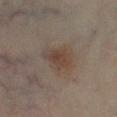Q: Was a biopsy performed?
A: catalogued during a skin exam; not biopsied
Q: How large is the lesion?
A: ≈3.5 mm
Q: Automated lesion metrics?
A: a lesion–skin lightness drop of about 6 and a normalized border contrast of about 6.5
Q: What kind of image is this?
A: 15 mm crop, total-body photography
Q: What lighting was used for the tile?
A: cross-polarized
Q: What is the anatomic site?
A: the leg
Q: Who is the patient?
A: female, aged around 60